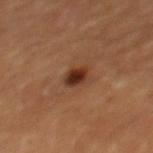Part of a total-body skin-imaging series; this lesion was reviewed on a skin check and was not flagged for biopsy.
The lesion is on the back.
Cropped from a whole-body photographic skin survey; the tile spans about 15 mm.
An algorithmic analysis of the crop reported a border-irregularity rating of about 1.5/10 and a color-variation rating of about 4.5/10. It also reported an automated nevus-likeness rating near 100 out of 100.
Longest diameter approximately 2.5 mm.
The subject is a male roughly 55 years of age.